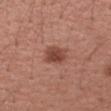{
  "biopsy_status": "not biopsied; imaged during a skin examination",
  "lesion_size": {
    "long_diameter_mm_approx": 3.5
  },
  "lighting": "white-light",
  "patient": {
    "sex": "female",
    "age_approx": 55
  },
  "site": "left upper arm",
  "image": {
    "source": "total-body photography crop",
    "field_of_view_mm": 15
  }
}